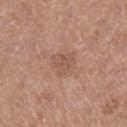No biopsy was performed on this lesion — it was imaged during a full skin examination and was not determined to be concerning. A female patient, about 40 years old. The tile uses white-light illumination. The total-body-photography lesion software estimated an average lesion color of about L≈54 a*≈21 b*≈28 (CIELAB), a lesion–skin lightness drop of about 7, and a normalized border contrast of about 5. And it measured a border-irregularity index near 3/10 and peripheral color asymmetry of about 1. And it measured a detector confidence of about 100 out of 100 that the crop contains a lesion. A 15 mm crop from a total-body photograph taken for skin-cancer surveillance. The lesion is on the right lower leg. Measured at roughly 3.5 mm in maximum diameter.